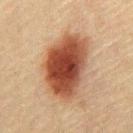body site: the chest
subject: male, aged 58 to 62
acquisition: total-body-photography crop, ~15 mm field of view
diameter: ~8.5 mm (longest diameter)